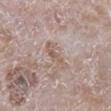patient:
  sex: male
  age_approx: 65
image:
  source: total-body photography crop
  field_of_view_mm: 15
site: right lower leg